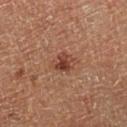<lesion>
  <biopsy_status>not biopsied; imaged during a skin examination</biopsy_status>
  <lesion_size>
    <long_diameter_mm_approx>3.0</long_diameter_mm_approx>
  </lesion_size>
  <site>leg</site>
  <patient>
    <sex>female</sex>
  </patient>
  <lighting>cross-polarized</lighting>
  <image>
    <source>total-body photography crop</source>
    <field_of_view_mm>15</field_of_view_mm>
  </image>
  <automated_metrics>
    <cielab_L>45</cielab_L>
    <cielab_a>24</cielab_a>
    <cielab_b>30</cielab_b>
    <vs_skin_darker_L>10.0</vs_skin_darker_L>
    <vs_skin_contrast_norm>7.5</vs_skin_contrast_norm>
  </automated_metrics>
</lesion>